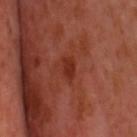Impression: Captured during whole-body skin photography for melanoma surveillance; the lesion was not biopsied. Acquisition and patient details: A male subject in their mid-50s. A 15 mm close-up tile from a total-body photography series done for melanoma screening. The lesion's longest dimension is about 2.5 mm. Automated image analysis of the tile measured a lesion area of about 3.5 mm², a shape eccentricity near 0.8, and a symmetry-axis asymmetry near 0.35. Located on the head or neck. This is a cross-polarized tile.